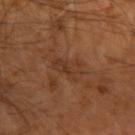No biopsy was performed on this lesion — it was imaged during a full skin examination and was not determined to be concerning. Automated tile analysis of the lesion measured a footprint of about 6 mm² and a shape eccentricity near 0.8. The software also gave an average lesion color of about L≈34 a*≈20 b*≈30 (CIELAB) and about 6 CIELAB-L* units darker than the surrounding skin. The patient is a male aged 58 to 62. The tile uses cross-polarized illumination. The recorded lesion diameter is about 3.5 mm. From the left arm. A 15 mm close-up extracted from a 3D total-body photography capture.